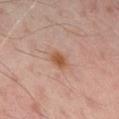| feature | finding |
|---|---|
| anatomic site | the back |
| image source | ~15 mm tile from a whole-body skin photo |
| illumination | cross-polarized illumination |
| automated metrics | a mean CIELAB color near L≈52 a*≈23 b*≈32, about 10 CIELAB-L* units darker than the surrounding skin, and a normalized lesion–skin contrast near 9; border irregularity of about 1.5 on a 0–10 scale, internal color variation of about 1.5 on a 0–10 scale, and peripheral color asymmetry of about 0.5 |
| diameter | ≈2 mm |
| subject | male, about 50 years old |